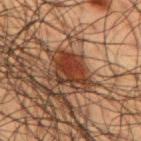Assessment: The lesion was tiled from a total-body skin photograph and was not biopsied. Acquisition and patient details: A male patient in their 60s. Captured under cross-polarized illumination. The lesion is located on the upper back. Cropped from a total-body skin-imaging series; the visible field is about 15 mm.